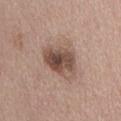| feature | finding |
|---|---|
| workup | catalogued during a skin exam; not biopsied |
| site | the front of the torso |
| subject | male, aged approximately 60 |
| acquisition | 15 mm crop, total-body photography |